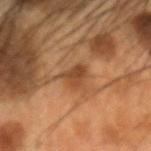No biopsy was performed on this lesion — it was imaged during a full skin examination and was not determined to be concerning. The lesion is located on the head or neck. A close-up tile cropped from a whole-body skin photograph, about 15 mm across. About 3 mm across. Automated tile analysis of the lesion measured internal color variation of about 3.5 on a 0–10 scale. The analysis additionally found a classifier nevus-likeness of about 30/100 and a detector confidence of about 95 out of 100 that the crop contains a lesion. A male subject, aged 53 to 57. The tile uses cross-polarized illumination.